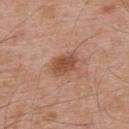follow-up: total-body-photography surveillance lesion; no biopsy